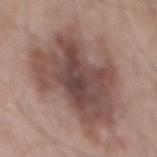workup: imaged on a skin check; not biopsied | image-analysis metrics: an area of roughly 55 mm², a shape eccentricity near 0.6, and a shape-asymmetry score of about 0.35 (0 = symmetric); a border-irregularity index near 5.5/10 and radial color variation of about 1.5 | tile lighting: white-light | subject: male, aged approximately 55 | image source: 15 mm crop, total-body photography | anatomic site: the mid back | lesion diameter: ~11 mm (longest diameter).